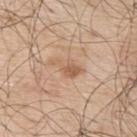workup=total-body-photography surveillance lesion; no biopsy
site=the back
TBP lesion metrics=radial color variation of about 0.5; lesion-presence confidence of about 100/100
patient=male, in their 70s
image source=~15 mm tile from a whole-body skin photo
diameter=≈4 mm
lighting=white-light illumination